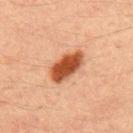Q: Was this lesion biopsied?
A: total-body-photography surveillance lesion; no biopsy
Q: What is the anatomic site?
A: the upper back
Q: What lighting was used for the tile?
A: cross-polarized illumination
Q: Patient demographics?
A: male, aged 28–32
Q: Lesion size?
A: ~5 mm (longest diameter)
Q: What is the imaging modality?
A: 15 mm crop, total-body photography
Q: What did automated image analysis measure?
A: internal color variation of about 3 on a 0–10 scale and radial color variation of about 1